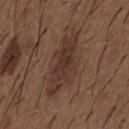follow-up=total-body-photography surveillance lesion; no biopsy
anatomic site=the chest
illumination=white-light illumination
imaging modality=15 mm crop, total-body photography
automated lesion analysis=a border-irregularity index near 3.5/10 and radial color variation of about 1.5
subject=male, aged 48 to 52